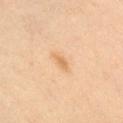Clinical impression: Captured during whole-body skin photography for melanoma surveillance; the lesion was not biopsied. Background: The subject is a male in their mid- to late 40s. Imaged with cross-polarized lighting. The lesion's longest dimension is about 2.5 mm. A close-up tile cropped from a whole-body skin photograph, about 15 mm across. Located on the chest. Automated image analysis of the tile measured a lesion area of about 2.5 mm², an eccentricity of roughly 0.85, and a shape-asymmetry score of about 0.4 (0 = symmetric). And it measured a mean CIELAB color near L≈68 a*≈20 b*≈40, about 8 CIELAB-L* units darker than the surrounding skin, and a lesion-to-skin contrast of about 6 (normalized; higher = more distinct). It also reported a classifier nevus-likeness of about 35/100.